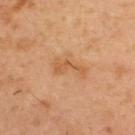follow-up — no biopsy performed (imaged during a skin exam)
site — the upper back
image source — ~15 mm tile from a whole-body skin photo
subject — male, in their mid- to late 50s